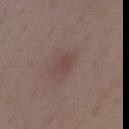Assessment: Recorded during total-body skin imaging; not selected for excision or biopsy. Clinical summary: The lesion is located on the mid back. A female subject aged 33 to 37. Cropped from a whole-body photographic skin survey; the tile spans about 15 mm.